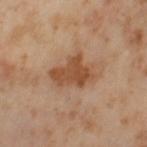The lesion was tiled from a total-body skin photograph and was not biopsied. The lesion's longest dimension is about 4 mm. Located on the left thigh. A female patient, approximately 55 years of age. A region of skin cropped from a whole-body photographic capture, roughly 15 mm wide. This is a cross-polarized tile.